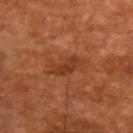| feature | finding |
|---|---|
| subject | male, roughly 65 years of age |
| tile lighting | cross-polarized illumination |
| image | 15 mm crop, total-body photography |
| diameter | ~3.5 mm (longest diameter) |